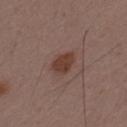Part of a total-body skin-imaging series; this lesion was reviewed on a skin check and was not flagged for biopsy.
A male subject, in their 50s.
The tile uses white-light illumination.
Located on the upper back.
A 15 mm crop from a total-body photograph taken for skin-cancer surveillance.
The lesion's longest dimension is about 3.5 mm.